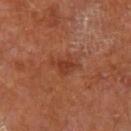Recorded during total-body skin imaging; not selected for excision or biopsy. The tile uses cross-polarized illumination. Approximately 3 mm at its widest. The patient is a male in their mid-60s. A 15 mm close-up tile from a total-body photography series done for melanoma screening. From the right lower leg.